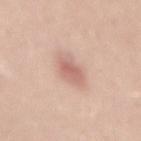{"biopsy_status": "not biopsied; imaged during a skin examination", "lesion_size": {"long_diameter_mm_approx": 4.0}, "lighting": "white-light", "image": {"source": "total-body photography crop", "field_of_view_mm": 15}, "automated_metrics": {"eccentricity": 0.9, "shape_asymmetry": 0.15, "cielab_L": 64, "cielab_a": 21, "cielab_b": 26, "vs_skin_contrast_norm": 6.5}, "patient": {"sex": "female", "age_approx": 35}, "site": "abdomen"}